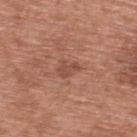| field | value |
|---|---|
| workup | imaged on a skin check; not biopsied |
| lesion size | ~2.5 mm (longest diameter) |
| tile lighting | white-light illumination |
| subject | male, approximately 70 years of age |
| automated lesion analysis | a footprint of about 4 mm², an outline eccentricity of about 0.7 (0 = round, 1 = elongated), and a symmetry-axis asymmetry near 0.3; a mean CIELAB color near L≈49 a*≈23 b*≈28, roughly 7 lightness units darker than nearby skin, and a lesion-to-skin contrast of about 5.5 (normalized; higher = more distinct) |
| site | the upper back |
| acquisition | 15 mm crop, total-body photography |